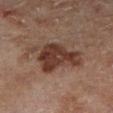| field | value |
|---|---|
| notes | no biopsy performed (imaged during a skin exam) |
| patient | male, aged around 65 |
| diameter | ~6 mm (longest diameter) |
| TBP lesion metrics | an eccentricity of roughly 0.7; an average lesion color of about L≈35 a*≈19 b*≈24 (CIELAB) and roughly 11 lightness units darker than nearby skin; a within-lesion color-variation index near 4.5/10 and peripheral color asymmetry of about 1.5 |
| image | total-body-photography crop, ~15 mm field of view |
| anatomic site | the right lower leg |